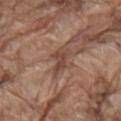Imaged during a routine full-body skin examination; the lesion was not biopsied and no histopathology is available.
The lesion is on the mid back.
A male subject, approximately 80 years of age.
A lesion tile, about 15 mm wide, cut from a 3D total-body photograph.
The tile uses white-light illumination.
Measured at roughly 3 mm in maximum diameter.